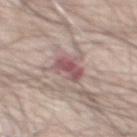notes: imaged on a skin check; not biopsied
body site: the front of the torso
subject: male, aged 58 to 62
tile lighting: white-light
image: 15 mm crop, total-body photography
lesion diameter: ~4 mm (longest diameter)
TBP lesion metrics: a lesion area of about 7 mm² and an outline eccentricity of about 0.7 (0 = round, 1 = elongated); a mean CIELAB color near L≈54 a*≈21 b*≈18, a lesion–skin lightness drop of about 12, and a normalized border contrast of about 8.5; lesion-presence confidence of about 100/100